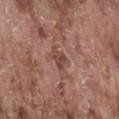Recorded during total-body skin imaging; not selected for excision or biopsy. The total-body-photography lesion software estimated a shape eccentricity near 0.8 and two-axis asymmetry of about 0.4. And it measured about 9 CIELAB-L* units darker than the surrounding skin and a normalized lesion–skin contrast near 6.5. The lesion is on the lower back. A male patient aged approximately 75. A roughly 15 mm field-of-view crop from a total-body skin photograph. Measured at roughly 3 mm in maximum diameter. This is a white-light tile.